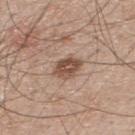{
  "biopsy_status": "not biopsied; imaged during a skin examination",
  "patient": {
    "sex": "male",
    "age_approx": 50
  },
  "lighting": "white-light",
  "site": "upper back",
  "image": {
    "source": "total-body photography crop",
    "field_of_view_mm": 15
  }
}